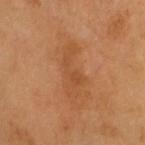Q: Was this lesion biopsied?
A: imaged on a skin check; not biopsied
Q: Lesion location?
A: the head or neck
Q: What lighting was used for the tile?
A: cross-polarized
Q: What is the imaging modality?
A: 15 mm crop, total-body photography
Q: Who is the patient?
A: male, approximately 60 years of age
Q: What did automated image analysis measure?
A: an area of roughly 9 mm² and two-axis asymmetry of about 0.45; a lesion color around L≈46 a*≈22 b*≈36 in CIELAB and a lesion-to-skin contrast of about 5 (normalized; higher = more distinct); a classifier nevus-likeness of about 0/100 and a detector confidence of about 100 out of 100 that the crop contains a lesion
Q: How large is the lesion?
A: ~5.5 mm (longest diameter)